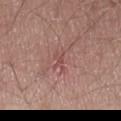biopsy status=no biopsy performed (imaged during a skin exam) | subject=male, aged 53–57 | illumination=white-light | anatomic site=the lower back | TBP lesion metrics=an average lesion color of about L≈50 a*≈24 b*≈23 (CIELAB) and a normalized border contrast of about 5; a classifier nevus-likeness of about 0/100 and a detector confidence of about 90 out of 100 that the crop contains a lesion | lesion size=~3 mm (longest diameter) | acquisition=total-body-photography crop, ~15 mm field of view.